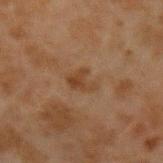Case summary:
* body site · the left forearm
* lesion diameter · about 3 mm
* acquisition · total-body-photography crop, ~15 mm field of view
* TBP lesion metrics · a lesion area of about 4.5 mm² and an outline eccentricity of about 0.65 (0 = round, 1 = elongated); a lesion color around L≈34 a*≈16 b*≈27 in CIELAB and a normalized lesion–skin contrast near 6.5; a color-variation rating of about 2.5/10 and a peripheral color-asymmetry measure near 0.5; a classifier nevus-likeness of about 10/100 and lesion-presence confidence of about 100/100
* patient · male, aged 43–47
* tile lighting · cross-polarized